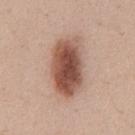Q: Is there a histopathology result?
A: no biopsy performed (imaged during a skin exam)
Q: Patient demographics?
A: male, aged 33 to 37
Q: How was the tile lit?
A: white-light
Q: Lesion location?
A: the abdomen
Q: What is the imaging modality?
A: total-body-photography crop, ~15 mm field of view